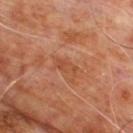Q: Is there a histopathology result?
A: imaged on a skin check; not biopsied
Q: Patient demographics?
A: male, about 60 years old
Q: What kind of image is this?
A: total-body-photography crop, ~15 mm field of view
Q: What is the anatomic site?
A: the back
Q: Illumination type?
A: cross-polarized